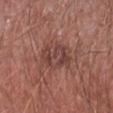biopsy_status: not biopsied; imaged during a skin examination
patient:
  sex: male
  age_approx: 65
site: left forearm
image:
  source: total-body photography crop
  field_of_view_mm: 15
lighting: white-light
automated_metrics:
  cielab_L: 42
  cielab_a: 22
  cielab_b: 23
  vs_skin_darker_L: 8.0
  vs_skin_contrast_norm: 6.5
  nevus_likeness_0_100: 0
  lesion_detection_confidence_0_100: 100